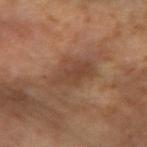workup = no biopsy performed (imaged during a skin exam)
illumination = cross-polarized
image = ~15 mm tile from a whole-body skin photo
patient = female, aged 48 to 52
site = the right forearm